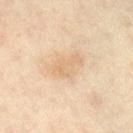Assessment: This lesion was catalogued during total-body skin photography and was not selected for biopsy. Acquisition and patient details: The lesion is located on the right lower leg. A female patient, in their mid- to late 40s. A region of skin cropped from a whole-body photographic capture, roughly 15 mm wide. The total-body-photography lesion software estimated a lesion area of about 7 mm² and a shape-asymmetry score of about 0.3 (0 = symmetric). It also reported an average lesion color of about L≈62 a*≈14 b*≈32 (CIELAB), about 6 CIELAB-L* units darker than the surrounding skin, and a normalized lesion–skin contrast near 5. The software also gave a border-irregularity rating of about 4/10 and radial color variation of about 0.5.